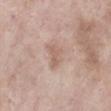The tile uses white-light illumination. Measured at roughly 3 mm in maximum diameter. A 15 mm close-up tile from a total-body photography series done for melanoma screening. A female patient, roughly 70 years of age. From the left lower leg.